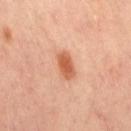<case>
<biopsy_status>not biopsied; imaged during a skin examination</biopsy_status>
<image>
  <source>total-body photography crop</source>
  <field_of_view_mm>15</field_of_view_mm>
</image>
<site>lower back</site>
<automated_metrics>
  <area_mm2_approx>5.5</area_mm2_approx>
  <shape_asymmetry>0.1</shape_asymmetry>
  <border_irregularity_0_10>1.5</border_irregularity_0_10>
  <color_variation_0_10>3.0</color_variation_0_10>
  <peripheral_color_asymmetry>1.0</peripheral_color_asymmetry>
</automated_metrics>
<lesion_size>
  <long_diameter_mm_approx>3.5</long_diameter_mm_approx>
</lesion_size>
<patient>
  <sex>female</sex>
  <age_approx>65</age_approx>
</patient>
</case>